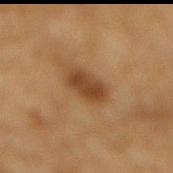The lesion was tiled from a total-body skin photograph and was not biopsied.
A close-up tile cropped from a whole-body skin photograph, about 15 mm across.
The lesion is on the back.
A male patient aged approximately 85 or older.
Automated tile analysis of the lesion measured an eccentricity of roughly 0.8 and two-axis asymmetry of about 0.2.